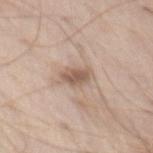<record>
<biopsy_status>not biopsied; imaged during a skin examination</biopsy_status>
<site>abdomen</site>
<lesion_size>
  <long_diameter_mm_approx>3.0</long_diameter_mm_approx>
</lesion_size>
<image>
  <source>total-body photography crop</source>
  <field_of_view_mm>15</field_of_view_mm>
</image>
<automated_metrics>
  <area_mm2_approx>5.5</area_mm2_approx>
  <eccentricity>0.65</eccentricity>
  <shape_asymmetry>0.25</shape_asymmetry>
  <cielab_L>57</cielab_L>
  <cielab_a>16</cielab_a>
  <cielab_b>26</cielab_b>
  <vs_skin_darker_L>11.0</vs_skin_darker_L>
  <vs_skin_contrast_norm>7.5</vs_skin_contrast_norm>
  <peripheral_color_asymmetry>1.0</peripheral_color_asymmetry>
</automated_metrics>
<patient>
  <sex>male</sex>
  <age_approx>40</age_approx>
</patient>
<lighting>white-light</lighting>
</record>